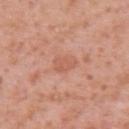{"biopsy_status": "not biopsied; imaged during a skin examination", "image": {"source": "total-body photography crop", "field_of_view_mm": 15}, "patient": {"sex": "female", "age_approx": 40}, "lighting": "white-light", "automated_metrics": {"eccentricity": 0.8, "shape_asymmetry": 0.2, "cielab_L": 59, "cielab_a": 26, "cielab_b": 32, "border_irregularity_0_10": 2.0, "nevus_likeness_0_100": 0, "lesion_detection_confidence_0_100": 100}, "site": "right upper arm", "lesion_size": {"long_diameter_mm_approx": 3.0}}